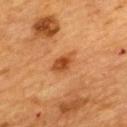follow-up=no biopsy performed (imaged during a skin exam)
lesion diameter=~3.5 mm (longest diameter)
subject=female, aged approximately 55
anatomic site=the upper back
acquisition=~15 mm crop, total-body skin-cancer survey
automated lesion analysis=a lesion area of about 5 mm² and a symmetry-axis asymmetry near 0.2; a mean CIELAB color near L≈41 a*≈24 b*≈36, roughly 9 lightness units darker than nearby skin, and a normalized lesion–skin contrast near 8; a color-variation rating of about 3/10
tile lighting=cross-polarized